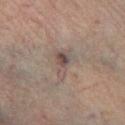Impression:
Captured during whole-body skin photography for melanoma surveillance; the lesion was not biopsied.
Context:
A 15 mm crop from a total-body photograph taken for skin-cancer surveillance. The lesion-visualizer software estimated a border-irregularity rating of about 5/10, a color-variation rating of about 6/10, and peripheral color asymmetry of about 2. It also reported a classifier nevus-likeness of about 5/100. The lesion's longest dimension is about 3.5 mm. The patient is a male aged 53 to 57. This is a cross-polarized tile. From the right lower leg.